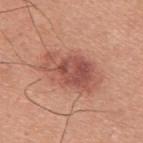Assessment: Imaged during a routine full-body skin examination; the lesion was not biopsied and no histopathology is available. Clinical summary: A male subject, aged approximately 30. About 6.5 mm across. The lesion is located on the upper back. A region of skin cropped from a whole-body photographic capture, roughly 15 mm wide. Automated image analysis of the tile measured a footprint of about 17 mm², an eccentricity of roughly 0.8, and two-axis asymmetry of about 0.3. The analysis additionally found a mean CIELAB color near L≈52 a*≈26 b*≈28, roughly 11 lightness units darker than nearby skin, and a normalized lesion–skin contrast near 8. The tile uses white-light illumination.